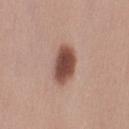Imaged during a routine full-body skin examination; the lesion was not biopsied and no histopathology is available.
The lesion's longest dimension is about 5 mm.
A region of skin cropped from a whole-body photographic capture, roughly 15 mm wide.
The lesion is located on the left thigh.
Captured under white-light illumination.
A female subject, in their mid- to late 30s.